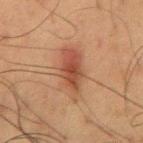follow-up — catalogued during a skin exam; not biopsied
lighting — cross-polarized illumination
image-analysis metrics — a footprint of about 13 mm² and a shape eccentricity near 0.9; a mean CIELAB color near L≈39 a*≈20 b*≈26, roughly 8 lightness units darker than nearby skin, and a lesion-to-skin contrast of about 7.5 (normalized; higher = more distinct); an automated nevus-likeness rating near 90 out of 100
patient — male, in their 60s
acquisition — ~15 mm tile from a whole-body skin photo
lesion diameter — ~6.5 mm (longest diameter)
anatomic site — the back